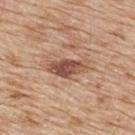workup — imaged on a skin check; not biopsied | tile lighting — white-light illumination | body site — the upper back | imaging modality — ~15 mm crop, total-body skin-cancer survey | patient — male, roughly 70 years of age | lesion diameter — about 5 mm | automated metrics — border irregularity of about 3.5 on a 0–10 scale, internal color variation of about 5 on a 0–10 scale, and peripheral color asymmetry of about 1.5; a nevus-likeness score of about 35/100.